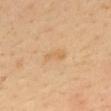| field | value |
|---|---|
| follow-up | total-body-photography surveillance lesion; no biopsy |
| image | total-body-photography crop, ~15 mm field of view |
| subject | male, aged 48–52 |
| location | the back |
| lighting | cross-polarized |